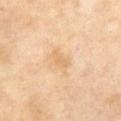follow-up: no biopsy performed (imaged during a skin exam)
anatomic site: the abdomen
patient: female, in their 50s
acquisition: ~15 mm crop, total-body skin-cancer survey
lighting: cross-polarized illumination